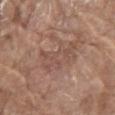patient = male, roughly 80 years of age
image source = 15 mm crop, total-body photography
location = the abdomen
size = about 5 mm
illumination = white-light
TBP lesion metrics = an average lesion color of about L≈50 a*≈19 b*≈25 (CIELAB); a border-irregularity index near 9/10 and a color-variation rating of about 2.5/10